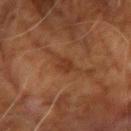This lesion was catalogued during total-body skin photography and was not selected for biopsy. The recorded lesion diameter is about 2.5 mm. A male subject about 70 years old. The lesion is located on the right upper arm. This is a cross-polarized tile. Cropped from a total-body skin-imaging series; the visible field is about 15 mm.